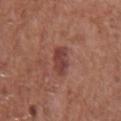biopsy status: catalogued during a skin exam; not biopsied
automated lesion analysis: an area of roughly 5.5 mm², an eccentricity of roughly 0.8, and a shape-asymmetry score of about 0.2 (0 = symmetric); roughly 10 lightness units darker than nearby skin and a lesion-to-skin contrast of about 8 (normalized; higher = more distinct); a border-irregularity rating of about 2.5/10, a within-lesion color-variation index near 2.5/10, and a peripheral color-asymmetry measure near 1; a classifier nevus-likeness of about 25/100 and a lesion-detection confidence of about 100/100
patient: male, in their mid- to late 70s
location: the front of the torso
diameter: about 3 mm
image source: total-body-photography crop, ~15 mm field of view